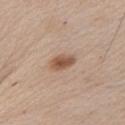– notes · imaged on a skin check; not biopsied
– subject · male, aged around 65
– site · the chest
– diameter · ≈3 mm
– tile lighting · white-light
– imaging modality · total-body-photography crop, ~15 mm field of view